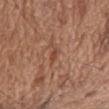Assessment: No biopsy was performed on this lesion — it was imaged during a full skin examination and was not determined to be concerning.